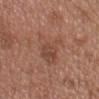Recorded during total-body skin imaging; not selected for excision or biopsy. A male patient, aged approximately 55. Imaged with white-light lighting. Located on the chest. This image is a 15 mm lesion crop taken from a total-body photograph.